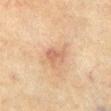Imaged during a routine full-body skin examination; the lesion was not biopsied and no histopathology is available. A close-up tile cropped from a whole-body skin photograph, about 15 mm across. The patient is a male in their mid-70s. Longest diameter approximately 2.5 mm. An algorithmic analysis of the crop reported an average lesion color of about L≈52 a*≈19 b*≈28 (CIELAB), roughly 8 lightness units darker than nearby skin, and a normalized border contrast of about 5.5. And it measured a border-irregularity index near 3/10 and internal color variation of about 1.5 on a 0–10 scale. The software also gave a classifier nevus-likeness of about 5/100 and a detector confidence of about 100 out of 100 that the crop contains a lesion. Located on the abdomen. Imaged with cross-polarized lighting.